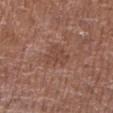Clinical impression: Captured during whole-body skin photography for melanoma surveillance; the lesion was not biopsied. Acquisition and patient details: A lesion tile, about 15 mm wide, cut from a 3D total-body photograph. The lesion is on the left lower leg. Imaged with white-light lighting. A female subject aged 78 to 82. Longest diameter approximately 3.5 mm. Automated tile analysis of the lesion measured a nevus-likeness score of about 0/100.